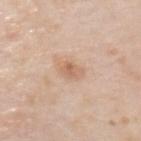Q: Lesion location?
A: the left upper arm
Q: How was this image acquired?
A: total-body-photography crop, ~15 mm field of view
Q: Who is the patient?
A: male, aged 73 to 77